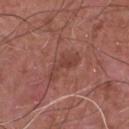notes: catalogued during a skin exam; not biopsied | image-analysis metrics: a footprint of about 6 mm² and a shape eccentricity near 0.95; an average lesion color of about L≈42 a*≈25 b*≈26 (CIELAB) and a normalized border contrast of about 5.5; a border-irregularity rating of about 6/10 and a within-lesion color-variation index near 1.5/10; an automated nevus-likeness rating near 0 out of 100 | lesion diameter: about 4.5 mm | imaging modality: total-body-photography crop, ~15 mm field of view | body site: the chest | patient: male, aged approximately 65.